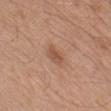{"biopsy_status": "not biopsied; imaged during a skin examination", "patient": {"sex": "male", "age_approx": 50}, "image": {"source": "total-body photography crop", "field_of_view_mm": 15}, "lesion_size": {"long_diameter_mm_approx": 3.0}, "site": "left forearm", "lighting": "white-light"}